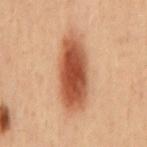Notes:
- workup — no biopsy performed (imaged during a skin exam)